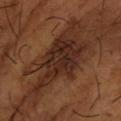workup — imaged on a skin check; not biopsied | location — the left forearm | tile lighting — cross-polarized | diameter — ~8.5 mm (longest diameter) | acquisition — ~15 mm tile from a whole-body skin photo | patient — male, in their mid- to late 60s.